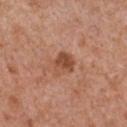biopsy status=imaged on a skin check; not biopsied | lighting=white-light | patient=male, in their mid- to late 60s | site=the chest | lesion diameter=about 3 mm | image=~15 mm tile from a whole-body skin photo.